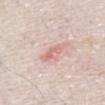Captured during whole-body skin photography for melanoma surveillance; the lesion was not biopsied. Measured at roughly 3 mm in maximum diameter. An algorithmic analysis of the crop reported a lesion area of about 3.5 mm² and two-axis asymmetry of about 0.35. And it measured an automated nevus-likeness rating near 0 out of 100. The patient is a male roughly 75 years of age. Located on the front of the torso. A 15 mm close-up extracted from a 3D total-body photography capture. The tile uses white-light illumination.